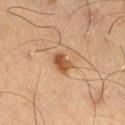subject = male, roughly 70 years of age
body site = the right thigh
illumination = cross-polarized illumination
acquisition = ~15 mm crop, total-body skin-cancer survey